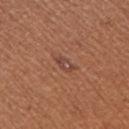Impression:
Part of a total-body skin-imaging series; this lesion was reviewed on a skin check and was not flagged for biopsy.
Background:
A female subject aged 53 to 57. A lesion tile, about 15 mm wide, cut from a 3D total-body photograph. Measured at roughly 2.5 mm in maximum diameter. The lesion-visualizer software estimated a nevus-likeness score of about 30/100 and a lesion-detection confidence of about 100/100. On the right upper arm. Captured under white-light illumination.